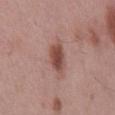Clinical impression: The lesion was tiled from a total-body skin photograph and was not biopsied. Image and clinical context: The lesion is on the mid back. Measured at roughly 4 mm in maximum diameter. The lesion-visualizer software estimated a lesion area of about 7.5 mm², a shape eccentricity near 0.8, and two-axis asymmetry of about 0.25. It also reported a mean CIELAB color near L≈48 a*≈22 b*≈24, about 12 CIELAB-L* units darker than the surrounding skin, and a normalized lesion–skin contrast near 8.5. Cropped from a total-body skin-imaging series; the visible field is about 15 mm. The tile uses white-light illumination. The subject is a male roughly 55 years of age.